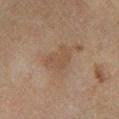workup = no biopsy performed (imaged during a skin exam)
acquisition = ~15 mm tile from a whole-body skin photo
patient = male, aged approximately 60
tile lighting = cross-polarized
size = ~3.5 mm (longest diameter)
location = the left leg
image-analysis metrics = a mean CIELAB color near L≈36 a*≈13 b*≈22, about 5 CIELAB-L* units darker than the surrounding skin, and a normalized border contrast of about 5; a border-irregularity rating of about 4/10 and internal color variation of about 1.5 on a 0–10 scale; a classifier nevus-likeness of about 0/100 and lesion-presence confidence of about 100/100